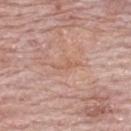Captured during whole-body skin photography for melanoma surveillance; the lesion was not biopsied.
The lesion is located on the upper back.
The recorded lesion diameter is about 3 mm.
A 15 mm crop from a total-body photograph taken for skin-cancer surveillance.
The total-body-photography lesion software estimated a lesion–skin lightness drop of about 6 and a lesion-to-skin contrast of about 4.5 (normalized; higher = more distinct). The software also gave border irregularity of about 7 on a 0–10 scale and internal color variation of about 0 on a 0–10 scale.
A female patient in their mid- to late 60s.